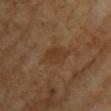| feature | finding |
|---|---|
| workup | imaged on a skin check; not biopsied |
| subject | female, approximately 70 years of age |
| diameter | ~3.5 mm (longest diameter) |
| tile lighting | cross-polarized |
| anatomic site | the chest |
| image | total-body-photography crop, ~15 mm field of view |